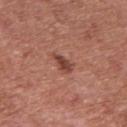<case>
<site>chest</site>
<lighting>white-light</lighting>
<lesion_size>
  <long_diameter_mm_approx>3.0</long_diameter_mm_approx>
</lesion_size>
<patient>
  <sex>male</sex>
  <age_approx>75</age_approx>
</patient>
<image>
  <source>total-body photography crop</source>
  <field_of_view_mm>15</field_of_view_mm>
</image>
</case>